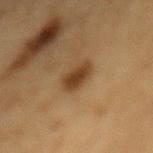<record>
<biopsy_status>not biopsied; imaged during a skin examination</biopsy_status>
<lesion_size>
  <long_diameter_mm_approx>3.5</long_diameter_mm_approx>
</lesion_size>
<patient>
  <sex>male</sex>
  <age_approx>85+</age_approx>
</patient>
<automated_metrics>
  <area_mm2_approx>5.5</area_mm2_approx>
  <eccentricity>0.8</eccentricity>
  <cielab_L>35</cielab_L>
  <cielab_a>16</cielab_a>
  <cielab_b>31</cielab_b>
  <vs_skin_darker_L>11.0</vs_skin_darker_L>
  <border_irregularity_0_10>2.5</border_irregularity_0_10>
  <color_variation_0_10>2.5</color_variation_0_10>
</automated_metrics>
<site>mid back</site>
<image>
  <source>total-body photography crop</source>
  <field_of_view_mm>15</field_of_view_mm>
</image>
</record>